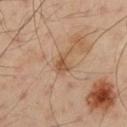| field | value |
|---|---|
| image source | ~15 mm tile from a whole-body skin photo |
| patient | male, aged 48–52 |
| automated lesion analysis | a mean CIELAB color near L≈52 a*≈19 b*≈32, a lesion–skin lightness drop of about 8, and a normalized lesion–skin contrast near 6.5; a border-irregularity rating of about 5/10; a classifier nevus-likeness of about 0/100 |
| tile lighting | cross-polarized |
| site | the left upper arm |